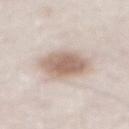No biopsy was performed on this lesion — it was imaged during a full skin examination and was not determined to be concerning. The lesion's longest dimension is about 4.5 mm. The tile uses white-light illumination. The lesion is located on the abdomen. The lesion-visualizer software estimated an area of roughly 16 mm² and a shape-asymmetry score of about 0.1 (0 = symmetric). It also reported a mean CIELAB color near L≈64 a*≈14 b*≈25, about 13 CIELAB-L* units darker than the surrounding skin, and a normalized border contrast of about 8. It also reported a color-variation rating of about 4/10 and a peripheral color-asymmetry measure near 1. A male patient aged 78–82. This image is a 15 mm lesion crop taken from a total-body photograph.